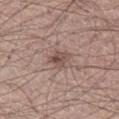The lesion was photographed on a routine skin check and not biopsied; there is no pathology result.
The total-body-photography lesion software estimated an average lesion color of about L≈49 a*≈17 b*≈23 (CIELAB) and a lesion–skin lightness drop of about 10. The software also gave a within-lesion color-variation index near 5/10. The analysis additionally found lesion-presence confidence of about 100/100.
Cropped from a whole-body photographic skin survey; the tile spans about 15 mm.
The tile uses white-light illumination.
Measured at roughly 3 mm in maximum diameter.
A male subject aged around 65.
The lesion is on the leg.